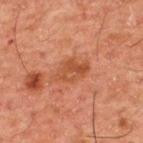This lesion was catalogued during total-body skin photography and was not selected for biopsy. A male subject aged approximately 50. This is a cross-polarized tile. Measured at roughly 4.5 mm in maximum diameter. The lesion is located on the upper back. A roughly 15 mm field-of-view crop from a total-body skin photograph.